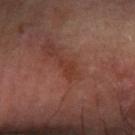biopsy status — imaged on a skin check; not biopsied.